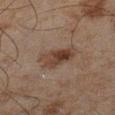The lesion was tiled from a total-body skin photograph and was not biopsied. Captured under cross-polarized illumination. Cropped from a total-body skin-imaging series; the visible field is about 15 mm. Located on the left lower leg. A male subject, aged around 45. The recorded lesion diameter is about 4 mm.